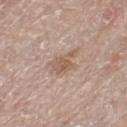Assessment: The lesion was photographed on a routine skin check and not biopsied; there is no pathology result. Context: A 15 mm close-up tile from a total-body photography series done for melanoma screening. A female patient aged approximately 60. From the right lower leg.